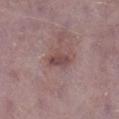The lesion was tiled from a total-body skin photograph and was not biopsied. A lesion tile, about 15 mm wide, cut from a 3D total-body photograph. Approximately 3 mm at its widest. The tile uses white-light illumination. A male patient, aged approximately 75. On the right lower leg. The lesion-visualizer software estimated a mean CIELAB color near L≈46 a*≈19 b*≈19 and a lesion-to-skin contrast of about 7 (normalized; higher = more distinct). And it measured a border-irregularity index near 3/10, a color-variation rating of about 2.5/10, and radial color variation of about 1.